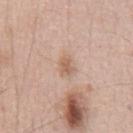The lesion was tiled from a total-body skin photograph and was not biopsied. Approximately 2.5 mm at its widest. Cropped from a whole-body photographic skin survey; the tile spans about 15 mm. The tile uses white-light illumination. Automated image analysis of the tile measured a lesion area of about 4 mm². The analysis additionally found a classifier nevus-likeness of about 0/100. A male patient, aged around 55. From the chest.